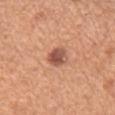| field | value |
|---|---|
| follow-up | imaged on a skin check; not biopsied |
| imaging modality | 15 mm crop, total-body photography |
| illumination | white-light |
| lesion size | ≈3 mm |
| body site | the mid back |
| automated lesion analysis | an automated nevus-likeness rating near 65 out of 100 and lesion-presence confidence of about 100/100 |
| patient | male, aged approximately 65 |